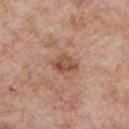Findings:
– workup: no biopsy performed (imaged during a skin exam)
– image: 15 mm crop, total-body photography
– location: the front of the torso
– diameter: ~3 mm (longest diameter)
– patient: male, aged around 60